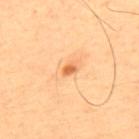Part of a total-body skin-imaging series; this lesion was reviewed on a skin check and was not flagged for biopsy.
Cropped from a whole-body photographic skin survey; the tile spans about 15 mm.
The recorded lesion diameter is about 2 mm.
A male subject in their mid- to late 60s.
The tile uses cross-polarized illumination.
On the mid back.